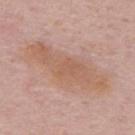image source: ~15 mm crop, total-body skin-cancer survey
patient: male, about 50 years old
automated lesion analysis: a lesion area of about 26 mm², a shape eccentricity near 0.95, and a shape-asymmetry score of about 0.25 (0 = symmetric); an average lesion color of about L≈59 a*≈20 b*≈29 (CIELAB), a lesion–skin lightness drop of about 7, and a lesion-to-skin contrast of about 6 (normalized; higher = more distinct); an automated nevus-likeness rating near 0 out of 100 and a detector confidence of about 100 out of 100 that the crop contains a lesion
anatomic site: the upper back
lighting: white-light
diameter: ≈10 mm